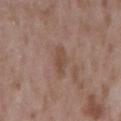Imaged during a routine full-body skin examination; the lesion was not biopsied and no histopathology is available. A male patient aged 48 to 52. Measured at roughly 2.5 mm in maximum diameter. A roughly 15 mm field-of-view crop from a total-body skin photograph. Imaged with white-light lighting. The lesion is located on the arm.